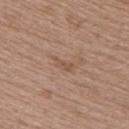biopsy status: no biopsy performed (imaged during a skin exam)
patient: female, about 60 years old
TBP lesion metrics: an eccentricity of roughly 0.9 and a symmetry-axis asymmetry near 0.35; internal color variation of about 0 on a 0–10 scale
anatomic site: the upper back
lighting: white-light illumination
imaging modality: ~15 mm crop, total-body skin-cancer survey
lesion size: ≈3 mm